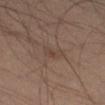biopsy status — catalogued during a skin exam; not biopsied
image-analysis metrics — a footprint of about 3.5 mm², a shape eccentricity near 0.75, and a shape-asymmetry score of about 0.35 (0 = symmetric)
location — the left leg
diameter — ≈3 mm
image — 15 mm crop, total-body photography
patient — male, aged 33–37